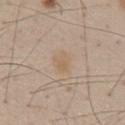follow-up: imaged on a skin check; not biopsied | tile lighting: white-light illumination | body site: the abdomen | automated metrics: an area of roughly 4 mm², an eccentricity of roughly 0.7, and a shape-asymmetry score of about 0.35 (0 = symmetric) | patient: male, aged approximately 45 | image source: ~15 mm tile from a whole-body skin photo.